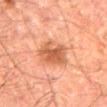Recorded during total-body skin imaging; not selected for excision or biopsy.
Automated image analysis of the tile measured a footprint of about 11 mm² and a shape eccentricity near 0.5. The software also gave an average lesion color of about L≈45 a*≈23 b*≈31 (CIELAB) and a lesion-to-skin contrast of about 7.5 (normalized; higher = more distinct). It also reported a border-irregularity rating of about 3/10, a within-lesion color-variation index near 3/10, and radial color variation of about 1. The analysis additionally found a nevus-likeness score of about 70/100 and lesion-presence confidence of about 100/100.
A 15 mm close-up extracted from a 3D total-body photography capture.
From the back.
The tile uses cross-polarized illumination.
The recorded lesion diameter is about 4 mm.
The patient is a male roughly 60 years of age.